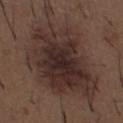Assessment:
The lesion was tiled from a total-body skin photograph and was not biopsied.
Image and clinical context:
A region of skin cropped from a whole-body photographic capture, roughly 15 mm wide. A male patient, aged around 50. The lesion's longest dimension is about 12 mm. The lesion is located on the chest. The tile uses white-light illumination.